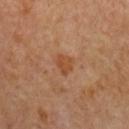follow-up = total-body-photography surveillance lesion; no biopsy
site = the chest
patient = female, in their mid- to late 60s
lighting = cross-polarized illumination
imaging modality = ~15 mm crop, total-body skin-cancer survey
automated metrics = a border-irregularity rating of about 3/10 and radial color variation of about 0.5
lesion diameter = ≈2.5 mm